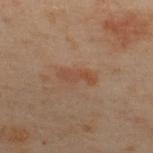Q: How was this image acquired?
A: ~15 mm tile from a whole-body skin photo
Q: What are the patient's age and sex?
A: male, in their 50s
Q: What is the anatomic site?
A: the upper back
Q: What lighting was used for the tile?
A: cross-polarized
Q: What is the lesion's diameter?
A: about 4 mm
Q: Automated lesion metrics?
A: border irregularity of about 4 on a 0–10 scale, a within-lesion color-variation index near 0.5/10, and a peripheral color-asymmetry measure near 0; a nevus-likeness score of about 15/100 and lesion-presence confidence of about 100/100